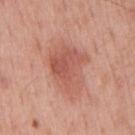Assessment: Recorded during total-body skin imaging; not selected for excision or biopsy. Image and clinical context: A 15 mm close-up extracted from a 3D total-body photography capture. This is a white-light tile. The lesion-visualizer software estimated an eccentricity of roughly 0.75 and two-axis asymmetry of about 0.25. The analysis additionally found a lesion–skin lightness drop of about 10 and a lesion-to-skin contrast of about 6.5 (normalized; higher = more distinct). And it measured peripheral color asymmetry of about 1.5. It also reported a classifier nevus-likeness of about 25/100. Longest diameter approximately 6 mm. A male subject approximately 55 years of age. The lesion is on the back.